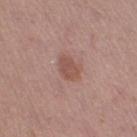Impression: Imaged during a routine full-body skin examination; the lesion was not biopsied and no histopathology is available. Context: The patient is a female roughly 30 years of age. Located on the right thigh. The lesion-visualizer software estimated a lesion area of about 4.5 mm², an outline eccentricity of about 0.7 (0 = round, 1 = elongated), and a symmetry-axis asymmetry near 0.2. And it measured a lesion color around L≈51 a*≈22 b*≈25 in CIELAB and about 9 CIELAB-L* units darker than the surrounding skin. The analysis additionally found a border-irregularity rating of about 2/10 and a peripheral color-asymmetry measure near 0.5. And it measured a classifier nevus-likeness of about 35/100 and a detector confidence of about 100 out of 100 that the crop contains a lesion. A close-up tile cropped from a whole-body skin photograph, about 15 mm across. The recorded lesion diameter is about 2.5 mm. This is a white-light tile.